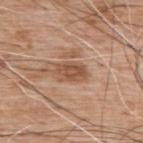{
  "biopsy_status": "not biopsied; imaged during a skin examination",
  "image": {
    "source": "total-body photography crop",
    "field_of_view_mm": 15
  },
  "site": "upper back",
  "patient": {
    "sex": "male",
    "age_approx": 60
  },
  "lesion_size": {
    "long_diameter_mm_approx": 3.5
  },
  "lighting": "white-light"
}